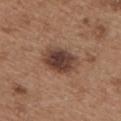biopsy_status: not biopsied; imaged during a skin examination
patient:
  sex: male
  age_approx: 65
image:
  source: total-body photography crop
  field_of_view_mm: 15
site: chest
lighting: white-light